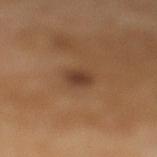• follow-up — total-body-photography surveillance lesion; no biopsy
• image — ~15 mm tile from a whole-body skin photo
• patient — male, about 65 years old
• anatomic site — the left lower leg
• diameter — about 2.5 mm
• tile lighting — cross-polarized illumination